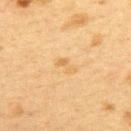The lesion is located on the upper back.
Automated image analysis of the tile measured a shape eccentricity near 0.9 and two-axis asymmetry of about 0.55. And it measured a lesion color around L≈57 a*≈16 b*≈39 in CIELAB, about 6 CIELAB-L* units darker than the surrounding skin, and a lesion-to-skin contrast of about 5.5 (normalized; higher = more distinct). The analysis additionally found internal color variation of about 0 on a 0–10 scale. And it measured a classifier nevus-likeness of about 0/100 and a lesion-detection confidence of about 100/100.
A roughly 15 mm field-of-view crop from a total-body skin photograph.
The tile uses cross-polarized illumination.
A female patient in their 40s.